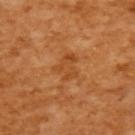Case summary:
– follow-up — imaged on a skin check; not biopsied
– subject — female, roughly 55 years of age
– anatomic site — the upper back
– lesion diameter — ≈3 mm
– imaging modality — 15 mm crop, total-body photography
– TBP lesion metrics — a lesion color around L≈49 a*≈27 b*≈44 in CIELAB, about 7 CIELAB-L* units darker than the surrounding skin, and a lesion-to-skin contrast of about 5.5 (normalized; higher = more distinct)
– tile lighting — cross-polarized illumination